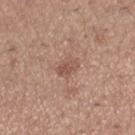Assessment: Part of a total-body skin-imaging series; this lesion was reviewed on a skin check and was not flagged for biopsy. Background: Captured under white-light illumination. Cropped from a total-body skin-imaging series; the visible field is about 15 mm. A female subject about 30 years old. On the right lower leg.